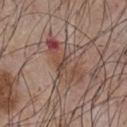The lesion was tiled from a total-body skin photograph and was not biopsied. The lesion is located on the chest. A male subject in their mid- to late 50s. Approximately 6.5 mm at its widest. A 15 mm close-up extracted from a 3D total-body photography capture.